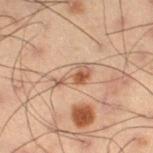biopsy status: imaged on a skin check; not biopsied
TBP lesion metrics: an outline eccentricity of about 0.9 (0 = round, 1 = elongated) and a symmetry-axis asymmetry near 0.55
image: ~15 mm crop, total-body skin-cancer survey
patient: male, aged around 55
lighting: cross-polarized
site: the leg
size: about 4 mm